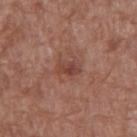<record>
  <biopsy_status>not biopsied; imaged during a skin examination</biopsy_status>
  <lesion_size>
    <long_diameter_mm_approx>3.0</long_diameter_mm_approx>
  </lesion_size>
  <site>abdomen</site>
  <image>
    <source>total-body photography crop</source>
    <field_of_view_mm>15</field_of_view_mm>
  </image>
  <patient>
    <sex>male</sex>
    <age_approx>75</age_approx>
  </patient>
</record>